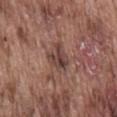No biopsy was performed on this lesion — it was imaged during a full skin examination and was not determined to be concerning.
Automated image analysis of the tile measured a border-irregularity rating of about 4/10, a within-lesion color-variation index near 6/10, and peripheral color asymmetry of about 2.5.
Measured at roughly 3 mm in maximum diameter.
From the lower back.
Cropped from a total-body skin-imaging series; the visible field is about 15 mm.
The subject is a male approximately 75 years of age.
The tile uses white-light illumination.